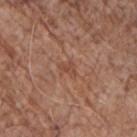The lesion was tiled from a total-body skin photograph and was not biopsied. Automated image analysis of the tile measured a footprint of about 3.5 mm², a shape eccentricity near 0.65, and a symmetry-axis asymmetry near 0.35. And it measured border irregularity of about 3.5 on a 0–10 scale, internal color variation of about 1.5 on a 0–10 scale, and a peripheral color-asymmetry measure near 0.5. The lesion is on the chest. The patient is a male aged 68–72. A region of skin cropped from a whole-body photographic capture, roughly 15 mm wide. About 2.5 mm across. This is a white-light tile.